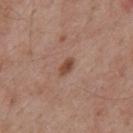- notes — total-body-photography surveillance lesion; no biopsy
- location — the back
- automated metrics — a lesion area of about 3 mm² and an eccentricity of roughly 0.85
- image — ~15 mm tile from a whole-body skin photo
- lesion diameter — ≈2.5 mm
- patient — male, aged 53 to 57